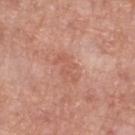<record>
<biopsy_status>not biopsied; imaged during a skin examination</biopsy_status>
<site>left lower leg</site>
<patient>
  <sex>female</sex>
  <age_approx>70</age_approx>
</patient>
<image>
  <source>total-body photography crop</source>
  <field_of_view_mm>15</field_of_view_mm>
</image>
<lighting>white-light</lighting>
</record>